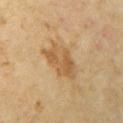{"biopsy_status": "not biopsied; imaged during a skin examination", "image": {"source": "total-body photography crop", "field_of_view_mm": 15}, "patient": {"sex": "female", "age_approx": 60}, "lighting": "cross-polarized", "lesion_size": {"long_diameter_mm_approx": 5.0}, "site": "left upper arm"}